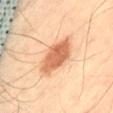The lesion was photographed on a routine skin check and not biopsied; there is no pathology result. This is a cross-polarized tile. A male subject approximately 65 years of age. On the abdomen. Approximately 6 mm at its widest. A 15 mm close-up tile from a total-body photography series done for melanoma screening.